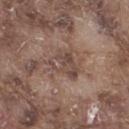Part of a total-body skin-imaging series; this lesion was reviewed on a skin check and was not flagged for biopsy.
Located on the right thigh.
Captured under white-light illumination.
A male subject approximately 75 years of age.
Cropped from a total-body skin-imaging series; the visible field is about 15 mm.